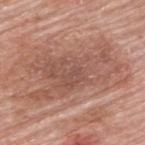Part of a total-body skin-imaging series; this lesion was reviewed on a skin check and was not flagged for biopsy.
Automated tile analysis of the lesion measured roughly 9 lightness units darker than nearby skin and a normalized lesion–skin contrast near 6. The software also gave a border-irregularity rating of about 8/10 and radial color variation of about 1.5.
This is a white-light tile.
From the upper back.
The recorded lesion diameter is about 10 mm.
A 15 mm close-up tile from a total-body photography series done for melanoma screening.
A male subject about 80 years old.